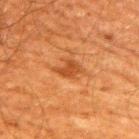Impression: This lesion was catalogued during total-body skin photography and was not selected for biopsy. Background: The lesion is on the upper back. The lesion's longest dimension is about 3.5 mm. A male patient approximately 60 years of age. A lesion tile, about 15 mm wide, cut from a 3D total-body photograph. Captured under cross-polarized illumination.